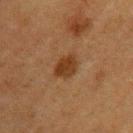The lesion was photographed on a routine skin check and not biopsied; there is no pathology result. The subject is a female aged around 55. Longest diameter approximately 3.5 mm. On the left upper arm. A roughly 15 mm field-of-view crop from a total-body skin photograph. An algorithmic analysis of the crop reported a lesion color around L≈31 a*≈18 b*≈30 in CIELAB, about 9 CIELAB-L* units darker than the surrounding skin, and a normalized lesion–skin contrast near 9. The software also gave a classifier nevus-likeness of about 100/100 and a detector confidence of about 100 out of 100 that the crop contains a lesion.